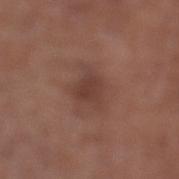biopsy status: total-body-photography surveillance lesion; no biopsy
site: the left lower leg
lesion diameter: about 3 mm
illumination: white-light
patient: female, aged around 80
TBP lesion metrics: a normalized lesion–skin contrast near 6.5; border irregularity of about 2.5 on a 0–10 scale and a within-lesion color-variation index near 1.5/10
image: ~15 mm tile from a whole-body skin photo